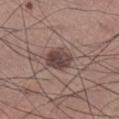Assessment:
Imaged during a routine full-body skin examination; the lesion was not biopsied and no histopathology is available.
Acquisition and patient details:
The total-body-photography lesion software estimated an eccentricity of roughly 0.6 and two-axis asymmetry of about 0.15. It also reported a lesion color around L≈43 a*≈17 b*≈19 in CIELAB, about 12 CIELAB-L* units darker than the surrounding skin, and a lesion-to-skin contrast of about 9.5 (normalized; higher = more distinct). And it measured border irregularity of about 1.5 on a 0–10 scale, internal color variation of about 4 on a 0–10 scale, and peripheral color asymmetry of about 1.5. The software also gave a nevus-likeness score of about 80/100. The subject is a male roughly 60 years of age. This is a white-light tile. From the right lower leg. Measured at roughly 3.5 mm in maximum diameter. A 15 mm close-up tile from a total-body photography series done for melanoma screening.